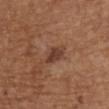Captured during whole-body skin photography for melanoma surveillance; the lesion was not biopsied. Automated tile analysis of the lesion measured a lesion area of about 5.5 mm², an eccentricity of roughly 0.8, and a shape-asymmetry score of about 0.25 (0 = symmetric). The software also gave a color-variation rating of about 3/10 and peripheral color asymmetry of about 1. The analysis additionally found a classifier nevus-likeness of about 45/100 and a detector confidence of about 100 out of 100 that the crop contains a lesion. A 15 mm close-up extracted from a 3D total-body photography capture. Longest diameter approximately 3 mm. The patient is a male in their 70s. The lesion is on the upper back.